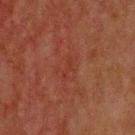Captured during whole-body skin photography for melanoma surveillance; the lesion was not biopsied.
A 15 mm crop from a total-body photograph taken for skin-cancer surveillance.
The tile uses cross-polarized illumination.
Approximately 3 mm at its widest.
The lesion-visualizer software estimated a lesion area of about 2.5 mm², an eccentricity of roughly 0.95, and two-axis asymmetry of about 0.5. It also reported a lesion color around L≈30 a*≈24 b*≈27 in CIELAB and a normalized border contrast of about 4.5. And it measured a border-irregularity index near 6/10 and a within-lesion color-variation index near 0/10. It also reported a classifier nevus-likeness of about 0/100 and a lesion-detection confidence of about 100/100.
On the upper back.
A male patient, roughly 70 years of age.